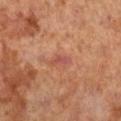Imaged during a routine full-body skin examination; the lesion was not biopsied and no histopathology is available. A female subject, roughly 65 years of age. An algorithmic analysis of the crop reported a border-irregularity rating of about 5/10, a within-lesion color-variation index near 0/10, and a peripheral color-asymmetry measure near 0. The analysis additionally found a nevus-likeness score of about 0/100 and a lesion-detection confidence of about 100/100. Located on the right lower leg. Cropped from a total-body skin-imaging series; the visible field is about 15 mm.